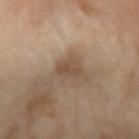Clinical impression: Part of a total-body skin-imaging series; this lesion was reviewed on a skin check and was not flagged for biopsy. Clinical summary: A lesion tile, about 15 mm wide, cut from a 3D total-body photograph. A female subject, aged approximately 70. Captured under cross-polarized illumination. The lesion's longest dimension is about 3.5 mm. From the left forearm.